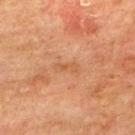workup: total-body-photography surveillance lesion; no biopsy
body site: the upper back
acquisition: 15 mm crop, total-body photography
illumination: cross-polarized
subject: male, aged 73 to 77
TBP lesion metrics: an area of roughly 2.5 mm², an outline eccentricity of about 0.95 (0 = round, 1 = elongated), and a symmetry-axis asymmetry near 0.3; a mean CIELAB color near L≈47 a*≈21 b*≈33, about 5 CIELAB-L* units darker than the surrounding skin, and a lesion-to-skin contrast of about 5 (normalized; higher = more distinct); a border-irregularity rating of about 4/10, a color-variation rating of about 0/10, and a peripheral color-asymmetry measure near 0; lesion-presence confidence of about 100/100